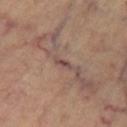• workup: catalogued during a skin exam; not biopsied